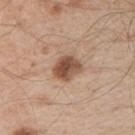The lesion was photographed on a routine skin check and not biopsied; there is no pathology result. Approximately 3.5 mm at its widest. Captured under white-light illumination. A 15 mm close-up tile from a total-body photography series done for melanoma screening. A male patient, aged 53–57. An algorithmic analysis of the crop reported an eccentricity of roughly 0.65 and a shape-asymmetry score of about 0.15 (0 = symmetric). It also reported an average lesion color of about L≈51 a*≈20 b*≈30 (CIELAB), about 15 CIELAB-L* units darker than the surrounding skin, and a normalized lesion–skin contrast near 10. The analysis additionally found a border-irregularity rating of about 1.5/10 and internal color variation of about 5 on a 0–10 scale. On the left upper arm.